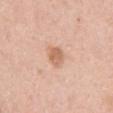<record>
<biopsy_status>not biopsied; imaged during a skin examination</biopsy_status>
<site>chest</site>
<image>
  <source>total-body photography crop</source>
  <field_of_view_mm>15</field_of_view_mm>
</image>
<patient>
  <sex>male</sex>
  <age_approx>50</age_approx>
</patient>
</record>